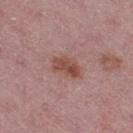notes: total-body-photography surveillance lesion; no biopsy | image source: ~15 mm crop, total-body skin-cancer survey | site: the right thigh | patient: female, aged approximately 45 | lesion size: about 3.5 mm | illumination: white-light illumination | image-analysis metrics: an average lesion color of about L≈48 a*≈23 b*≈24 (CIELAB) and a lesion–skin lightness drop of about 10; a border-irregularity rating of about 3/10, a color-variation rating of about 3.5/10, and a peripheral color-asymmetry measure near 1.5.